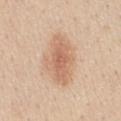This lesion was catalogued during total-body skin photography and was not selected for biopsy. This image is a 15 mm lesion crop taken from a total-body photograph. Imaged with white-light lighting. Measured at roughly 6.5 mm in maximum diameter. The subject is a male approximately 30 years of age. The lesion-visualizer software estimated border irregularity of about 2.5 on a 0–10 scale, a within-lesion color-variation index near 3.5/10, and radial color variation of about 1. It also reported a nevus-likeness score of about 80/100. On the chest.